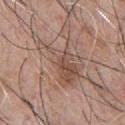workup: total-body-photography surveillance lesion; no biopsy | lesion diameter: ≈8 mm | site: the chest | illumination: white-light illumination | acquisition: total-body-photography crop, ~15 mm field of view | patient: male, aged around 45 | automated metrics: a mean CIELAB color near L≈52 a*≈17 b*≈25, roughly 9 lightness units darker than nearby skin, and a normalized lesion–skin contrast near 6; an automated nevus-likeness rating near 0 out of 100 and a detector confidence of about 95 out of 100 that the crop contains a lesion.